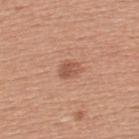The lesion was photographed on a routine skin check and not biopsied; there is no pathology result.
The lesion is located on the upper back.
A 15 mm crop from a total-body photograph taken for skin-cancer surveillance.
A female patient, aged approximately 45.
Automated tile analysis of the lesion measured an area of roughly 4.5 mm² and a symmetry-axis asymmetry near 0.25. It also reported an average lesion color of about L≈55 a*≈23 b*≈30 (CIELAB), about 9 CIELAB-L* units darker than the surrounding skin, and a lesion-to-skin contrast of about 6 (normalized; higher = more distinct). The analysis additionally found peripheral color asymmetry of about 1.